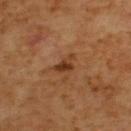Case summary:
• biopsy status · imaged on a skin check; not biopsied
• subject · male, about 65 years old
• diameter · ≈3 mm
• location · the upper back
• imaging modality · total-body-photography crop, ~15 mm field of view
• tile lighting · cross-polarized illumination